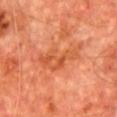Recorded during total-body skin imaging; not selected for excision or biopsy. The lesion is located on the chest. A male patient, approximately 65 years of age. About 5.5 mm across. A region of skin cropped from a whole-body photographic capture, roughly 15 mm wide. The tile uses cross-polarized illumination.